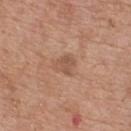Case summary:
• biopsy status: total-body-photography surveillance lesion; no biopsy
• lesion diameter: about 2.5 mm
• site: the upper back
• TBP lesion metrics: a shape eccentricity near 0.6 and a symmetry-axis asymmetry near 0.2; a classifier nevus-likeness of about 0/100 and lesion-presence confidence of about 100/100
• illumination: white-light
• subject: male, aged 78–82
• image: ~15 mm crop, total-body skin-cancer survey